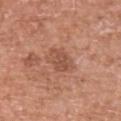{"biopsy_status": "not biopsied; imaged during a skin examination", "image": {"source": "total-body photography crop", "field_of_view_mm": 15}, "patient": {"sex": "male", "age_approx": 45}, "site": "upper back"}